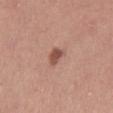Captured during whole-body skin photography for melanoma surveillance; the lesion was not biopsied. A roughly 15 mm field-of-view crop from a total-body skin photograph. The subject is a female aged 48–52. Captured under white-light illumination. Longest diameter approximately 3 mm. Automated tile analysis of the lesion measured an average lesion color of about L≈52 a*≈23 b*≈26 (CIELAB), a lesion–skin lightness drop of about 12, and a lesion-to-skin contrast of about 8.5 (normalized; higher = more distinct). On the leg.